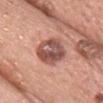Impression:
Captured during whole-body skin photography for melanoma surveillance; the lesion was not biopsied.
Acquisition and patient details:
Cropped from a total-body skin-imaging series; the visible field is about 15 mm. On the head or neck. A female subject aged around 60.